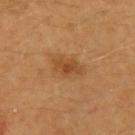• workup — catalogued during a skin exam; not biopsied
• image-analysis metrics — an average lesion color of about L≈47 a*≈22 b*≈40 (CIELAB), a lesion–skin lightness drop of about 9, and a normalized border contrast of about 7; a border-irregularity index near 4/10, a within-lesion color-variation index near 2/10, and peripheral color asymmetry of about 0.5
• patient — male, approximately 40 years of age
• tile lighting — cross-polarized illumination
• diameter — ≈3 mm
• image source — ~15 mm tile from a whole-body skin photo
• site — the left upper arm